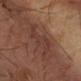follow-up: imaged on a skin check; not biopsied
body site: the left forearm
patient: male, aged around 60
image: total-body-photography crop, ~15 mm field of view